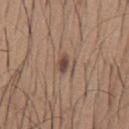Part of a total-body skin-imaging series; this lesion was reviewed on a skin check and was not flagged for biopsy. This is a white-light tile. The patient is a male roughly 65 years of age. Approximately 3 mm at its widest. From the chest. An algorithmic analysis of the crop reported an average lesion color of about L≈48 a*≈16 b*≈25 (CIELAB) and about 9 CIELAB-L* units darker than the surrounding skin. And it measured a border-irregularity index near 5/10, internal color variation of about 4 on a 0–10 scale, and radial color variation of about 1. And it measured an automated nevus-likeness rating near 80 out of 100. This image is a 15 mm lesion crop taken from a total-body photograph.